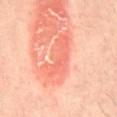No biopsy was performed on this lesion — it was imaged during a full skin examination and was not determined to be concerning. The patient is a male in their 40s. The lesion's longest dimension is about 5.5 mm. This is a cross-polarized tile. The lesion is located on the back. A 15 mm crop from a total-body photograph taken for skin-cancer surveillance.